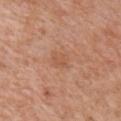Part of a total-body skin-imaging series; this lesion was reviewed on a skin check and was not flagged for biopsy. Imaged with white-light lighting. Longest diameter approximately 2.5 mm. A male patient about 60 years old. A lesion tile, about 15 mm wide, cut from a 3D total-body photograph. From the chest.